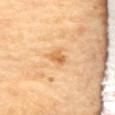Q: Is there a histopathology result?
A: imaged on a skin check; not biopsied
Q: How large is the lesion?
A: about 2.5 mm
Q: Where on the body is the lesion?
A: the upper back
Q: What kind of image is this?
A: ~15 mm crop, total-body skin-cancer survey
Q: What are the patient's age and sex?
A: female, aged around 70
Q: How was the tile lit?
A: cross-polarized